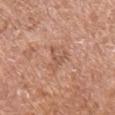On the arm. The recorded lesion diameter is about 4 mm. A 15 mm close-up tile from a total-body photography series done for melanoma screening. Imaged with white-light lighting. A male patient, aged around 65.